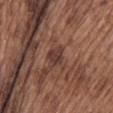<tbp_lesion>
<biopsy_status>not biopsied; imaged during a skin examination</biopsy_status>
<automated_metrics>
  <area_mm2_approx>4.5</area_mm2_approx>
  <eccentricity>0.45</eccentricity>
  <shape_asymmetry>0.35</shape_asymmetry>
  <cielab_L>37</cielab_L>
  <cielab_a>19</cielab_a>
  <cielab_b>23</cielab_b>
  <vs_skin_darker_L>9.0</vs_skin_darker_L>
  <vs_skin_contrast_norm>8.5</vs_skin_contrast_norm>
  <nevus_likeness_0_100>5</nevus_likeness_0_100>
</automated_metrics>
<lesion_size>
  <long_diameter_mm_approx>2.5</long_diameter_mm_approx>
</lesion_size>
<patient>
  <sex>male</sex>
  <age_approx>75</age_approx>
</patient>
<site>upper back</site>
<image>
  <source>total-body photography crop</source>
  <field_of_view_mm>15</field_of_view_mm>
</image>
<lighting>white-light</lighting>
</tbp_lesion>